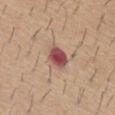subject — male, aged around 60
TBP lesion metrics — a footprint of about 6.5 mm² and a shape-asymmetry score of about 0.2 (0 = symmetric); a mean CIELAB color near L≈50 a*≈25 b*≈24, a lesion–skin lightness drop of about 15, and a normalized border contrast of about 11
tile lighting — white-light illumination
anatomic site — the front of the torso
image source — ~15 mm crop, total-body skin-cancer survey